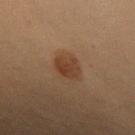biopsy_status: not biopsied; imaged during a skin examination
image:
  source: total-body photography crop
  field_of_view_mm: 15
lighting: cross-polarized
site: upper back
automated_metrics:
  area_mm2_approx: 6.5
  eccentricity: 0.7
  shape_asymmetry: 0.15
  border_irregularity_0_10: 1.5
  peripheral_color_asymmetry: 1.0
  lesion_detection_confidence_0_100: 100
lesion_size:
  long_diameter_mm_approx: 3.5
patient:
  sex: female
  age_approx: 45